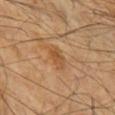– notes: total-body-photography surveillance lesion; no biopsy
– subject: male, aged 68–72
– imaging modality: total-body-photography crop, ~15 mm field of view
– anatomic site: the left forearm
– automated lesion analysis: a lesion–skin lightness drop of about 7; a border-irregularity rating of about 3/10 and a within-lesion color-variation index near 2.5/10; a nevus-likeness score of about 0/100 and a lesion-detection confidence of about 100/100